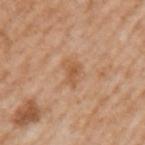workup — total-body-photography surveillance lesion; no biopsy | lighting — white-light | size — ~2.5 mm (longest diameter) | anatomic site — the left upper arm | subject — male, about 65 years old | acquisition — total-body-photography crop, ~15 mm field of view | image-analysis metrics — a lesion area of about 4 mm², a shape eccentricity near 0.75, and two-axis asymmetry of about 0.4; a border-irregularity index near 4/10, a color-variation rating of about 1.5/10, and radial color variation of about 0.5; an automated nevus-likeness rating near 0 out of 100 and a detector confidence of about 100 out of 100 that the crop contains a lesion.